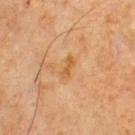The lesion was tiled from a total-body skin photograph and was not biopsied. A male patient, roughly 65 years of age. The lesion is located on the chest. A 15 mm close-up extracted from a 3D total-body photography capture.